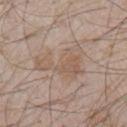The lesion was tiled from a total-body skin photograph and was not biopsied.
A male patient, in their mid-60s.
The tile uses white-light illumination.
From the chest.
An algorithmic analysis of the crop reported a shape eccentricity near 0.8. The analysis additionally found a mean CIELAB color near L≈56 a*≈15 b*≈27, a lesion–skin lightness drop of about 7, and a normalized border contrast of about 5.5. The software also gave an automated nevus-likeness rating near 0 out of 100 and a lesion-detection confidence of about 100/100.
Cropped from a whole-body photographic skin survey; the tile spans about 15 mm.